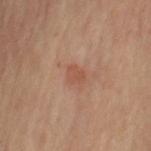Impression:
This lesion was catalogued during total-body skin photography and was not selected for biopsy.
Image and clinical context:
A female subject, aged 63–67. The lesion is located on the left upper arm. This is a cross-polarized tile. Cropped from a total-body skin-imaging series; the visible field is about 15 mm.